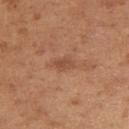workup — total-body-photography surveillance lesion; no biopsy
image — ~15 mm tile from a whole-body skin photo
automated metrics — a border-irregularity index near 4/10, internal color variation of about 1.5 on a 0–10 scale, and radial color variation of about 0.5; a classifier nevus-likeness of about 0/100 and a detector confidence of about 100 out of 100 that the crop contains a lesion
patient — female, roughly 30 years of age
site — the left upper arm